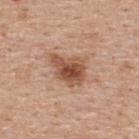patient = male, aged around 55; anatomic site = the upper back; acquisition = total-body-photography crop, ~15 mm field of view.